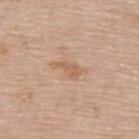The subject is a male aged around 75. From the upper back. The lesion's longest dimension is about 3.5 mm. Cropped from a total-body skin-imaging series; the visible field is about 15 mm. Automated tile analysis of the lesion measured a footprint of about 4.5 mm² and two-axis asymmetry of about 0.45. The analysis additionally found about 7 CIELAB-L* units darker than the surrounding skin and a normalized border contrast of about 6. The software also gave a classifier nevus-likeness of about 0/100 and a lesion-detection confidence of about 100/100. Captured under white-light illumination.